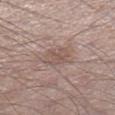Captured during whole-body skin photography for melanoma surveillance; the lesion was not biopsied. Cropped from a whole-body photographic skin survey; the tile spans about 15 mm. A male patient, roughly 55 years of age. Located on the left lower leg. Captured under white-light illumination.